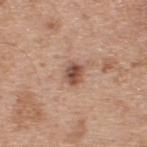Case summary:
- workup: imaged on a skin check; not biopsied
- size: ~2.5 mm (longest diameter)
- patient: male, approximately 55 years of age
- tile lighting: white-light
- acquisition: 15 mm crop, total-body photography
- site: the upper back
- image-analysis metrics: a mean CIELAB color near L≈51 a*≈21 b*≈28, a lesion–skin lightness drop of about 14, and a normalized lesion–skin contrast near 9.5; a border-irregularity index near 2/10, a within-lesion color-variation index near 5/10, and a peripheral color-asymmetry measure near 1.5